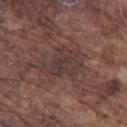workup = imaged on a skin check; not biopsied | automated metrics = a lesion area of about 12 mm², a shape eccentricity near 0.75, and a symmetry-axis asymmetry near 0.4; a within-lesion color-variation index near 3.5/10; an automated nevus-likeness rating near 0 out of 100 | subject = male, in their mid- to late 70s | acquisition = ~15 mm tile from a whole-body skin photo | illumination = white-light illumination | lesion diameter = about 5 mm | anatomic site = the left thigh.